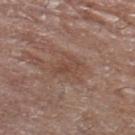A 15 mm crop from a total-body photograph taken for skin-cancer surveillance.
The lesion is on the right thigh.
A female patient about 75 years old.